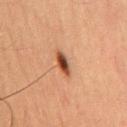Findings:
– biopsy status — no biopsy performed (imaged during a skin exam)
– illumination — cross-polarized illumination
– size — ~3.5 mm (longest diameter)
– acquisition — 15 mm crop, total-body photography
– patient — male, approximately 60 years of age
– image-analysis metrics — a normalized border contrast of about 10.5; a border-irregularity index near 3/10, a color-variation rating of about 8/10, and a peripheral color-asymmetry measure near 2.5